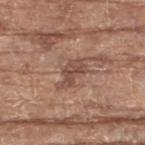follow-up — no biopsy performed (imaged during a skin exam); illumination — white-light illumination; anatomic site — the right thigh; acquisition — ~15 mm tile from a whole-body skin photo; subject — female, aged 73 to 77; lesion diameter — ~4 mm (longest diameter).